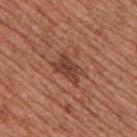lesion size: ~3.5 mm (longest diameter)
anatomic site: the upper back
image: ~15 mm tile from a whole-body skin photo
TBP lesion metrics: a lesion color around L≈42 a*≈25 b*≈27 in CIELAB, a lesion–skin lightness drop of about 10, and a lesion-to-skin contrast of about 7.5 (normalized; higher = more distinct); a border-irregularity rating of about 3.5/10 and peripheral color asymmetry of about 1
subject: male, aged around 55